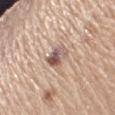Automated image analysis of the tile measured a lesion area of about 6 mm² and a symmetry-axis asymmetry near 0.25. And it measured a border-irregularity index near 3/10, a within-lesion color-variation index near 9.5/10, and peripheral color asymmetry of about 3.5. A female subject aged 63 to 67. The lesion is located on the right upper arm. Cropped from a whole-body photographic skin survey; the tile spans about 15 mm. Measured at roughly 3 mm in maximum diameter. Imaged with white-light lighting.